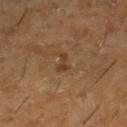| field | value |
|---|---|
| follow-up | total-body-photography surveillance lesion; no biopsy |
| anatomic site | the right lower leg |
| automated metrics | an eccentricity of roughly 0.9 and two-axis asymmetry of about 0.55; internal color variation of about 0 on a 0–10 scale and radial color variation of about 0 |
| tile lighting | cross-polarized |
| acquisition | 15 mm crop, total-body photography |
| size | ~2.5 mm (longest diameter) |
| patient | male, roughly 60 years of age |